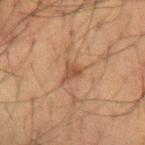This lesion was catalogued during total-body skin photography and was not selected for biopsy. A region of skin cropped from a whole-body photographic capture, roughly 15 mm wide. The lesion is on the left forearm. A male subject aged 58 to 62. Captured under cross-polarized illumination. Approximately 2.5 mm at its widest.